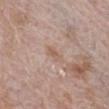Q: Where on the body is the lesion?
A: the right upper arm
Q: What are the patient's age and sex?
A: male, about 70 years old
Q: What is the imaging modality?
A: ~15 mm tile from a whole-body skin photo